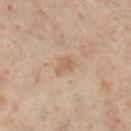biopsy status = total-body-photography surveillance lesion; no biopsy | tile lighting = cross-polarized illumination | location = the left leg | TBP lesion metrics = a footprint of about 4.5 mm², a shape eccentricity near 0.8, and a symmetry-axis asymmetry near 0.2; an automated nevus-likeness rating near 0 out of 100 and a detector confidence of about 100 out of 100 that the crop contains a lesion | patient = female, aged 53 to 57 | image = total-body-photography crop, ~15 mm field of view.